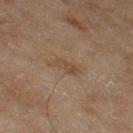Q: Was a biopsy performed?
A: imaged on a skin check; not biopsied
Q: What kind of image is this?
A: ~15 mm tile from a whole-body skin photo
Q: What are the patient's age and sex?
A: female, about 60 years old
Q: Lesion location?
A: the leg